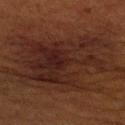notes: no biopsy performed (imaged during a skin exam).